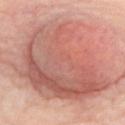workup — imaged on a skin check; not biopsied | acquisition — total-body-photography crop, ~15 mm field of view | lesion size — about 17 mm | image-analysis metrics — a lesion color around L≈60 a*≈25 b*≈27 in CIELAB and a lesion-to-skin contrast of about 8 (normalized; higher = more distinct); border irregularity of about 5.5 on a 0–10 scale, a color-variation rating of about 8.5/10, and a peripheral color-asymmetry measure near 3; a nevus-likeness score of about 65/100 and a lesion-detection confidence of about 90/100 | subject — female, roughly 75 years of age | tile lighting — cross-polarized | anatomic site — the upper back.